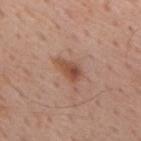<record>
  <biopsy_status>not biopsied; imaged during a skin examination</biopsy_status>
  <lesion_size>
    <long_diameter_mm_approx>3.5</long_diameter_mm_approx>
  </lesion_size>
  <site>mid back</site>
  <patient>
    <sex>male</sex>
    <age_approx>60</age_approx>
  </patient>
  <lighting>white-light</lighting>
  <image>
    <source>total-body photography crop</source>
    <field_of_view_mm>15</field_of_view_mm>
  </image>
</record>